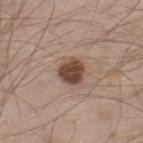Part of a total-body skin-imaging series; this lesion was reviewed on a skin check and was not flagged for biopsy. Approximately 3 mm at its widest. Cropped from a whole-body photographic skin survey; the tile spans about 15 mm. A male patient aged approximately 35. On the right lower leg. Automated image analysis of the tile measured border irregularity of about 1.5 on a 0–10 scale and a color-variation rating of about 4/10.